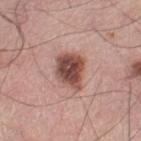No biopsy was performed on this lesion — it was imaged during a full skin examination and was not determined to be concerning. A 15 mm crop from a total-body photograph taken for skin-cancer surveillance. The lesion is located on the left thigh. The tile uses white-light illumination. A male patient, aged 68–72. The recorded lesion diameter is about 4.5 mm.